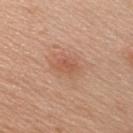Findings:
– notes: catalogued during a skin exam; not biopsied